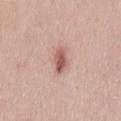Clinical impression:
Part of a total-body skin-imaging series; this lesion was reviewed on a skin check and was not flagged for biopsy.
Acquisition and patient details:
A male subject about 50 years old. A 15 mm close-up tile from a total-body photography series done for melanoma screening. On the left thigh.